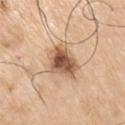Case summary:
- workup — imaged on a skin check; not biopsied
- anatomic site — the right upper arm
- subject — male, approximately 75 years of age
- size — ≈5 mm
- acquisition — ~15 mm tile from a whole-body skin photo
- lighting — white-light illumination